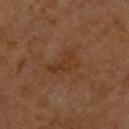* follow-up · no biopsy performed (imaged during a skin exam)
* location · the chest
* patient · male, roughly 60 years of age
* imaging modality · 15 mm crop, total-body photography